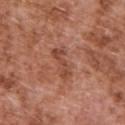Cropped from a total-body skin-imaging series; the visible field is about 15 mm. A male patient, aged around 75. The lesion is on the back. Captured under white-light illumination.